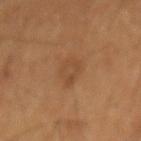Q: Was a biopsy performed?
A: catalogued during a skin exam; not biopsied
Q: How large is the lesion?
A: ~3.5 mm (longest diameter)
Q: What are the patient's age and sex?
A: male, in their 60s
Q: What did automated image analysis measure?
A: an area of roughly 6 mm², an eccentricity of roughly 0.8, and a symmetry-axis asymmetry near 0.25; a nevus-likeness score of about 0/100
Q: What is the imaging modality?
A: ~15 mm crop, total-body skin-cancer survey
Q: Where on the body is the lesion?
A: the mid back